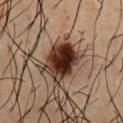No biopsy was performed on this lesion — it was imaged during a full skin examination and was not determined to be concerning.
Located on the chest.
A lesion tile, about 15 mm wide, cut from a 3D total-body photograph.
Captured under cross-polarized illumination.
A male subject, roughly 55 years of age.
Measured at roughly 5 mm in maximum diameter.